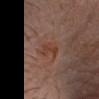The lesion was tiled from a total-body skin photograph and was not biopsied. Cropped from a whole-body photographic skin survey; the tile spans about 15 mm. On the head or neck. A male subject, about 65 years old. Automated image analysis of the tile measured a footprint of about 5.5 mm², an eccentricity of roughly 0.9, and a symmetry-axis asymmetry near 0.35. The software also gave a border-irregularity index near 3.5/10, a color-variation rating of about 2.5/10, and peripheral color asymmetry of about 0.5. And it measured an automated nevus-likeness rating near 0 out of 100.